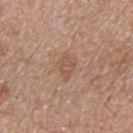Assessment:
No biopsy was performed on this lesion — it was imaged during a full skin examination and was not determined to be concerning.
Image and clinical context:
The lesion is on the right forearm. Imaged with white-light lighting. A close-up tile cropped from a whole-body skin photograph, about 15 mm across. The subject is a female aged 48–52. About 2.5 mm across. Automated tile analysis of the lesion measured an average lesion color of about L≈54 a*≈20 b*≈30 (CIELAB), roughly 7 lightness units darker than nearby skin, and a normalized lesion–skin contrast near 5.